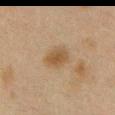Case summary:
– notes · catalogued during a skin exam; not biopsied
– acquisition · 15 mm crop, total-body photography
– patient · female, approximately 40 years of age
– tile lighting · cross-polarized
– lesion size · ≈3 mm
– body site · the chest